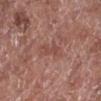workup=no biopsy performed (imaged during a skin exam) | lighting=white-light illumination | lesion size=about 4 mm | acquisition=~15 mm tile from a whole-body skin photo | site=the right lower leg | subject=male, about 75 years old | image-analysis metrics=a lesion area of about 5.5 mm² and an eccentricity of roughly 0.9; about 6 CIELAB-L* units darker than the surrounding skin; a detector confidence of about 100 out of 100 that the crop contains a lesion.